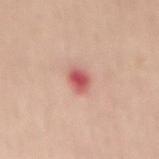{
  "biopsy_status": "not biopsied; imaged during a skin examination",
  "site": "mid back",
  "lesion_size": {
    "long_diameter_mm_approx": 2.5
  },
  "patient": {
    "sex": "female",
    "age_approx": 50
  },
  "image": {
    "source": "total-body photography crop",
    "field_of_view_mm": 15
  }
}